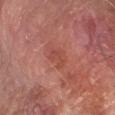Assessment:
This lesion was catalogued during total-body skin photography and was not selected for biopsy.
Image and clinical context:
Approximately 2.5 mm at its widest. A lesion tile, about 15 mm wide, cut from a 3D total-body photograph. The lesion is on the right forearm. A male patient, roughly 70 years of age. An algorithmic analysis of the crop reported a shape eccentricity near 0.75 and two-axis asymmetry of about 0.3. It also reported an average lesion color of about L≈46 a*≈28 b*≈28 (CIELAB), roughly 6 lightness units darker than nearby skin, and a lesion-to-skin contrast of about 5 (normalized; higher = more distinct). It also reported an automated nevus-likeness rating near 0 out of 100 and a detector confidence of about 100 out of 100 that the crop contains a lesion. This is a cross-polarized tile.